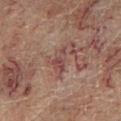Findings:
* biopsy status: total-body-photography surveillance lesion; no biopsy
* acquisition: total-body-photography crop, ~15 mm field of view
* anatomic site: the left lower leg
* subject: male, aged around 60
* automated lesion analysis: a footprint of about 3.5 mm² and an outline eccentricity of about 0.85 (0 = round, 1 = elongated); an automated nevus-likeness rating near 0 out of 100 and lesion-presence confidence of about 95/100